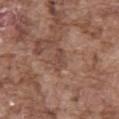{"biopsy_status": "not biopsied; imaged during a skin examination", "automated_metrics": {"area_mm2_approx": 3.0, "eccentricity": 0.9, "vs_skin_darker_L": 8.0, "vs_skin_contrast_norm": 6.0, "nevus_likeness_0_100": 0, "lesion_detection_confidence_0_100": 80}, "image": {"source": "total-body photography crop", "field_of_view_mm": 15}, "site": "front of the torso", "patient": {"sex": "male", "age_approx": 75}}